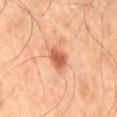Findings:
• biopsy status — no biopsy performed (imaged during a skin exam)
• lighting — cross-polarized illumination
• patient — male, roughly 55 years of age
• imaging modality — total-body-photography crop, ~15 mm field of view
• lesion diameter — about 3 mm
• automated lesion analysis — a footprint of about 5.5 mm², an eccentricity of roughly 0.65, and a symmetry-axis asymmetry near 0.25; a border-irregularity index near 2/10, a color-variation rating of about 2.5/10, and peripheral color asymmetry of about 1; a classifier nevus-likeness of about 95/100 and lesion-presence confidence of about 100/100
• site — the mid back